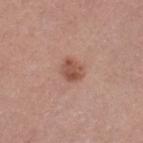Impression:
The lesion was photographed on a routine skin check and not biopsied; there is no pathology result.
Context:
About 2.5 mm across. The tile uses white-light illumination. A 15 mm close-up tile from a total-body photography series done for melanoma screening. The subject is a female aged 28 to 32. From the left thigh.